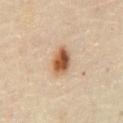Assessment:
No biopsy was performed on this lesion — it was imaged during a full skin examination and was not determined to be concerning.
Background:
A male patient aged 48 to 52. The recorded lesion diameter is about 4 mm. The tile uses cross-polarized illumination. A close-up tile cropped from a whole-body skin photograph, about 15 mm across. The lesion is on the abdomen.